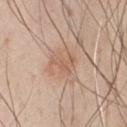biopsy status: imaged on a skin check; not biopsied
illumination: white-light
subject: male, approximately 45 years of age
size: ~3 mm (longest diameter)
location: the chest
image source: ~15 mm crop, total-body skin-cancer survey